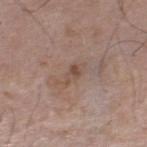biopsy status = no biopsy performed (imaged during a skin exam) | anatomic site = the leg | size = about 2.5 mm | tile lighting = white-light | imaging modality = total-body-photography crop, ~15 mm field of view | patient = male, roughly 70 years of age.